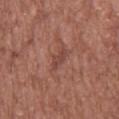The lesion was tiled from a total-body skin photograph and was not biopsied.
A region of skin cropped from a whole-body photographic capture, roughly 15 mm wide.
Measured at roughly 3 mm in maximum diameter.
Located on the upper back.
The patient is a male aged 58–62.